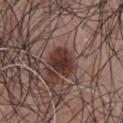Located on the chest. The subject is a male aged 68 to 72. The total-body-photography lesion software estimated a lesion area of about 7.5 mm² and two-axis asymmetry of about 0.2. A roughly 15 mm field-of-view crop from a total-body skin photograph. Captured under white-light illumination.